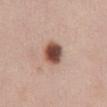The lesion was tiled from a total-body skin photograph and was not biopsied. The lesion is on the abdomen. A 15 mm close-up extracted from a 3D total-body photography capture. About 3.5 mm across. A female subject, aged approximately 50.